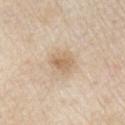Impression: The lesion was tiled from a total-body skin photograph and was not biopsied. Clinical summary: Longest diameter approximately 3 mm. On the right upper arm. A male subject aged around 80. The tile uses white-light illumination. The total-body-photography lesion software estimated a footprint of about 5 mm², an eccentricity of roughly 0.65, and two-axis asymmetry of about 0.3. The analysis additionally found a nevus-likeness score of about 20/100 and lesion-presence confidence of about 100/100. A 15 mm close-up tile from a total-body photography series done for melanoma screening.